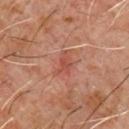A male subject, about 60 years old. A 15 mm close-up extracted from a 3D total-body photography capture. From the chest.